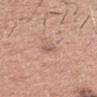Imaged with white-light lighting. Automated image analysis of the tile measured a lesion color around L≈58 a*≈19 b*≈25 in CIELAB, a lesion–skin lightness drop of about 8, and a normalized border contrast of about 5.5. The analysis additionally found a border-irregularity index near 3.5/10 and a within-lesion color-variation index near 1/10. A 15 mm close-up tile from a total-body photography series done for melanoma screening. The patient is a male approximately 30 years of age. The lesion is located on the head or neck. About 2.5 mm across.